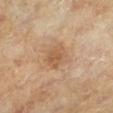Imaged during a routine full-body skin examination; the lesion was not biopsied and no histopathology is available. A male patient about 65 years old. Longest diameter approximately 3 mm. The tile uses cross-polarized illumination. This image is a 15 mm lesion crop taken from a total-body photograph. Located on the left lower leg. The lesion-visualizer software estimated a lesion color around L≈57 a*≈19 b*≈35 in CIELAB, a lesion–skin lightness drop of about 8, and a normalized lesion–skin contrast near 6. The analysis additionally found a border-irregularity index near 2/10, internal color variation of about 2.5 on a 0–10 scale, and radial color variation of about 0.5. It also reported a nevus-likeness score of about 40/100.